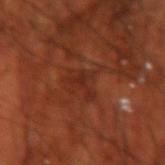diameter — ~4 mm (longest diameter) | automated lesion analysis — a lesion area of about 8.5 mm² and a symmetry-axis asymmetry near 0.35; border irregularity of about 5.5 on a 0–10 scale and a color-variation rating of about 3/10; a nevus-likeness score of about 0/100 and lesion-presence confidence of about 75/100 | site — the arm | acquisition — 15 mm crop, total-body photography | patient — male, about 70 years old.